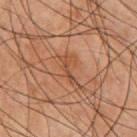  biopsy_status: not biopsied; imaged during a skin examination
  patient:
    sex: male
    age_approx: 70
  site: chest
  image:
    source: total-body photography crop
    field_of_view_mm: 15